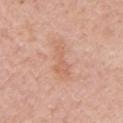Clinical impression: The lesion was tiled from a total-body skin photograph and was not biopsied. Clinical summary: Captured under white-light illumination. A female subject, aged 63 to 67. The total-body-photography lesion software estimated a footprint of about 8 mm², an eccentricity of roughly 0.9, and a shape-asymmetry score of about 0.4 (0 = symmetric). The software also gave a lesion color around L≈63 a*≈22 b*≈32 in CIELAB and roughly 6 lightness units darker than nearby skin. The analysis additionally found a classifier nevus-likeness of about 0/100 and a detector confidence of about 100 out of 100 that the crop contains a lesion. From the arm. Cropped from a total-body skin-imaging series; the visible field is about 15 mm. About 4.5 mm across.